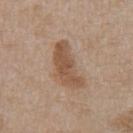Impression:
Imaged during a routine full-body skin examination; the lesion was not biopsied and no histopathology is available.